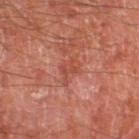Imaged during a routine full-body skin examination; the lesion was not biopsied and no histopathology is available. The tile uses cross-polarized illumination. A male subject roughly 70 years of age. The total-body-photography lesion software estimated a lesion color around L≈48 a*≈29 b*≈31 in CIELAB, a lesion–skin lightness drop of about 7, and a normalized lesion–skin contrast near 6. The analysis additionally found a border-irregularity index near 6/10, internal color variation of about 2 on a 0–10 scale, and peripheral color asymmetry of about 0.5. The software also gave an automated nevus-likeness rating near 0 out of 100 and a detector confidence of about 90 out of 100 that the crop contains a lesion. A 15 mm close-up extracted from a 3D total-body photography capture. On the left thigh. About 3 mm across.